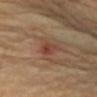Q: Is there a histopathology result?
A: total-body-photography surveillance lesion; no biopsy
Q: What is the imaging modality?
A: total-body-photography crop, ~15 mm field of view
Q: What did automated image analysis measure?
A: a lesion–skin lightness drop of about 8 and a normalized border contrast of about 6.5; a border-irregularity index near 2.5/10, internal color variation of about 5.5 on a 0–10 scale, and peripheral color asymmetry of about 2; a lesion-detection confidence of about 100/100
Q: Who is the patient?
A: in their 60s
Q: Lesion size?
A: ~2.5 mm (longest diameter)
Q: Lesion location?
A: the right upper arm
Q: Illumination type?
A: cross-polarized illumination